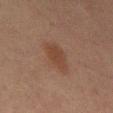Clinical impression:
Imaged during a routine full-body skin examination; the lesion was not biopsied and no histopathology is available.
Background:
The lesion is on the mid back. Automated tile analysis of the lesion measured a footprint of about 6.5 mm². It also reported about 6 CIELAB-L* units darker than the surrounding skin and a normalized lesion–skin contrast near 6.5. The tile uses cross-polarized illumination. A 15 mm close-up extracted from a 3D total-body photography capture. A male patient about 65 years old.